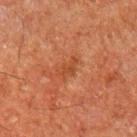Clinical impression:
Part of a total-body skin-imaging series; this lesion was reviewed on a skin check and was not flagged for biopsy.
Context:
Longest diameter approximately 3 mm. The subject is a male roughly 75 years of age. The tile uses cross-polarized illumination. A close-up tile cropped from a whole-body skin photograph, about 15 mm across. On the arm.